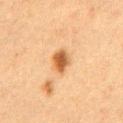workup=no biopsy performed (imaged during a skin exam) | lesion size=≈3.5 mm | image source=~15 mm crop, total-body skin-cancer survey | automated metrics=border irregularity of about 1.5 on a 0–10 scale, internal color variation of about 3.5 on a 0–10 scale, and peripheral color asymmetry of about 1; lesion-presence confidence of about 100/100 | body site=the chest | patient=male, roughly 50 years of age.